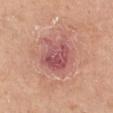Impression:
Recorded during total-body skin imaging; not selected for excision or biopsy.
Background:
The subject is a male aged around 55. Cropped from a whole-body photographic skin survey; the tile spans about 15 mm. Located on the right lower leg.